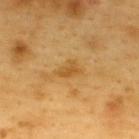Background: A male subject roughly 60 years of age. On the upper back. A close-up tile cropped from a whole-body skin photograph, about 15 mm across.